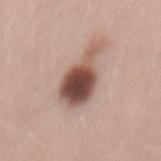  biopsy_status: not biopsied; imaged during a skin examination
  patient:
    sex: male
    age_approx: 30
  image:
    source: total-body photography crop
    field_of_view_mm: 15